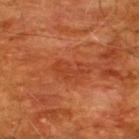{
  "biopsy_status": "not biopsied; imaged during a skin examination",
  "automated_metrics": {
    "area_mm2_approx": 5.5,
    "eccentricity": 0.9,
    "shape_asymmetry": 0.55,
    "cielab_L": 40,
    "cielab_a": 30,
    "cielab_b": 37,
    "vs_skin_darker_L": 6.0,
    "nevus_likeness_0_100": 0,
    "lesion_detection_confidence_0_100": 100
  },
  "patient": {
    "sex": "male",
    "age_approx": 60
  },
  "site": "back",
  "image": {
    "source": "total-body photography crop",
    "field_of_view_mm": 15
  },
  "lesion_size": {
    "long_diameter_mm_approx": 4.0
  },
  "lighting": "cross-polarized"
}